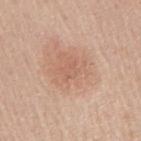Part of a total-body skin-imaging series; this lesion was reviewed on a skin check and was not flagged for biopsy. From the right upper arm. A 15 mm close-up extracted from a 3D total-body photography capture. This is a white-light tile. A male patient, about 45 years old. Approximately 2 mm at its widest.